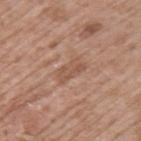Findings:
• workup · no biopsy performed (imaged during a skin exam)